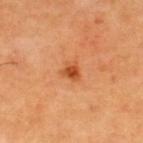Recorded during total-body skin imaging; not selected for excision or biopsy. This is a cross-polarized tile. Located on the back. The lesion-visualizer software estimated an area of roughly 3.5 mm², an eccentricity of roughly 0.4, and a shape-asymmetry score of about 0.35 (0 = symmetric). The analysis additionally found border irregularity of about 3.5 on a 0–10 scale and a color-variation rating of about 3/10. A male patient, aged 63 to 67. A roughly 15 mm field-of-view crop from a total-body skin photograph.